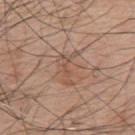Impression: The lesion was tiled from a total-body skin photograph and was not biopsied. Clinical summary: A male patient, aged around 75. The lesion is located on the mid back. A lesion tile, about 15 mm wide, cut from a 3D total-body photograph. The recorded lesion diameter is about 4 mm. Imaged with white-light lighting.